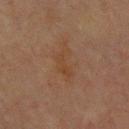Findings:
* biopsy status · no biopsy performed (imaged during a skin exam)
* acquisition · 15 mm crop, total-body photography
* size · about 4 mm
* lighting · cross-polarized illumination
* automated lesion analysis · a footprint of about 5.5 mm² and a shape-asymmetry score of about 0.6 (0 = symmetric); border irregularity of about 7 on a 0–10 scale, a color-variation rating of about 2/10, and a peripheral color-asymmetry measure near 0.5; an automated nevus-likeness rating near 0 out of 100 and a detector confidence of about 100 out of 100 that the crop contains a lesion
* anatomic site · the chest
* patient · male, aged 68–72